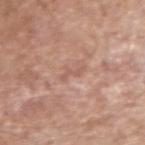Assessment:
No biopsy was performed on this lesion — it was imaged during a full skin examination and was not determined to be concerning.
Context:
Captured under white-light illumination. Longest diameter approximately 2.5 mm. The patient is a male about 65 years old. The lesion is located on the right upper arm. A region of skin cropped from a whole-body photographic capture, roughly 15 mm wide.